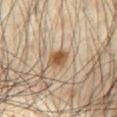Impression: The lesion was photographed on a routine skin check and not biopsied; there is no pathology result. Clinical summary: A roughly 15 mm field-of-view crop from a total-body skin photograph. The patient is a male aged approximately 60. The lesion is on the abdomen.